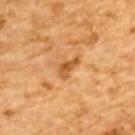Case summary:
• notes — catalogued during a skin exam; not biopsied
• location — the back
• subject — male, about 85 years old or older
• imaging modality — 15 mm crop, total-body photography